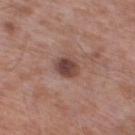<lesion>
<biopsy_status>not biopsied; imaged during a skin examination</biopsy_status>
<image>
  <source>total-body photography crop</source>
  <field_of_view_mm>15</field_of_view_mm>
</image>
<patient>
  <sex>male</sex>
  <age_approx>55</age_approx>
</patient>
<site>left lower leg</site>
</lesion>